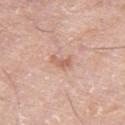Findings:
– imaging modality: ~15 mm crop, total-body skin-cancer survey
– body site: the leg
– patient: male, aged approximately 80